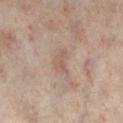workup: total-body-photography surveillance lesion; no biopsy | anatomic site: the right lower leg | subject: female, approximately 50 years of age | image source: 15 mm crop, total-body photography | size: ~3 mm (longest diameter) | automated metrics: a lesion area of about 3.5 mm², a shape eccentricity near 0.9, and a shape-asymmetry score of about 0.5 (0 = symmetric); an average lesion color of about L≈54 a*≈16 b*≈24 (CIELAB) and about 7 CIELAB-L* units darker than the surrounding skin; a border-irregularity index near 5.5/10, a color-variation rating of about 1/10, and radial color variation of about 0.5.